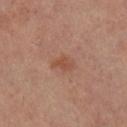Context: The patient is a female aged approximately 60. The lesion is located on the right lower leg. A 15 mm close-up extracted from a 3D total-body photography capture.